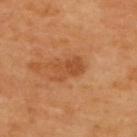workup=catalogued during a skin exam; not biopsied | location=the upper back | acquisition=15 mm crop, total-body photography | subject=aged approximately 70.